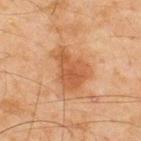Notes:
- biopsy status — no biopsy performed (imaged during a skin exam)
- subject — male, about 45 years old
- automated metrics — a mean CIELAB color near L≈45 a*≈21 b*≈33, a lesion–skin lightness drop of about 8, and a normalized lesion–skin contrast near 7; a border-irregularity rating of about 4.5/10, internal color variation of about 3 on a 0–10 scale, and radial color variation of about 1; an automated nevus-likeness rating near 25 out of 100 and lesion-presence confidence of about 100/100
- image — total-body-photography crop, ~15 mm field of view
- site — the upper back
- tile lighting — cross-polarized illumination
- size — about 5 mm